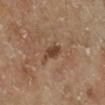Q: Was this lesion biopsied?
A: no biopsy performed (imaged during a skin exam)
Q: Automated lesion metrics?
A: a mean CIELAB color near L≈42 a*≈20 b*≈30, about 10 CIELAB-L* units darker than the surrounding skin, and a normalized lesion–skin contrast near 8.5; a color-variation rating of about 1/10 and peripheral color asymmetry of about 0.5; a classifier nevus-likeness of about 75/100
Q: What lighting was used for the tile?
A: cross-polarized
Q: What is the lesion's diameter?
A: ≈3 mm
Q: Where on the body is the lesion?
A: the right lower leg
Q: What kind of image is this?
A: ~15 mm crop, total-body skin-cancer survey
Q: Who is the patient?
A: male, aged 63 to 67